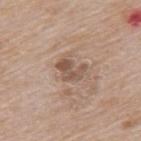This lesion was catalogued during total-body skin photography and was not selected for biopsy. A male subject roughly 65 years of age. From the upper back. A close-up tile cropped from a whole-body skin photograph, about 15 mm across.